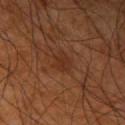| field | value |
|---|---|
| biopsy status | no biopsy performed (imaged during a skin exam) |
| size | ~2.5 mm (longest diameter) |
| image source | total-body-photography crop, ~15 mm field of view |
| illumination | cross-polarized |
| subject | male, aged 63 to 67 |
| automated lesion analysis | an outline eccentricity of about 0.55 (0 = round, 1 = elongated) and a symmetry-axis asymmetry near 0.25; a border-irregularity rating of about 2.5/10 and a color-variation rating of about 2/10 |
| site | the right upper arm |